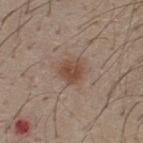The lesion was tiled from a total-body skin photograph and was not biopsied.
A region of skin cropped from a whole-body photographic capture, roughly 15 mm wide.
Captured under white-light illumination.
Longest diameter approximately 3 mm.
The lesion is on the upper back.
A male patient approximately 30 years of age.